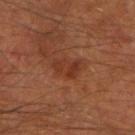| feature | finding |
|---|---|
| workup | catalogued during a skin exam; not biopsied |
| image | total-body-photography crop, ~15 mm field of view |
| body site | the arm |
| lesion diameter | ~3.5 mm (longest diameter) |
| patient | male, aged approximately 60 |
| image-analysis metrics | a footprint of about 6 mm², a shape eccentricity near 0.75, and two-axis asymmetry of about 0.35; a border-irregularity rating of about 4/10 and a color-variation rating of about 2.5/10; a nevus-likeness score of about 0/100 and a lesion-detection confidence of about 100/100 |